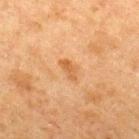acquisition: 15 mm crop, total-body photography | automated metrics: roughly 8 lightness units darker than nearby skin and a lesion-to-skin contrast of about 6.5 (normalized; higher = more distinct); a border-irregularity index near 3.5/10, internal color variation of about 0 on a 0–10 scale, and peripheral color asymmetry of about 0 | diameter: ≈2.5 mm | site: the upper back | patient: male, aged 48–52 | illumination: cross-polarized.